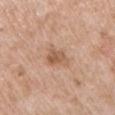Assessment: The lesion was photographed on a routine skin check and not biopsied; there is no pathology result. Context: The lesion is located on the chest. This image is a 15 mm lesion crop taken from a total-body photograph. The subject is a male aged 48 to 52. This is a white-light tile. Approximately 3.5 mm at its widest.